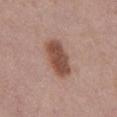biopsy_status: not biopsied; imaged during a skin examination
site: front of the torso
image:
  source: total-body photography crop
  field_of_view_mm: 15
patient:
  sex: male
  age_approx: 55
lighting: white-light
automated_metrics:
  cielab_L: 49
  cielab_a: 21
  cielab_b: 27
  vs_skin_contrast_norm: 10.0
  border_irregularity_0_10: 1.5
  color_variation_0_10: 2.5
  peripheral_color_asymmetry: 1.0
  nevus_likeness_0_100: 95
  lesion_detection_confidence_0_100: 100
lesion_size:
  long_diameter_mm_approx: 5.0